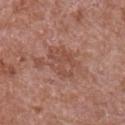Recorded during total-body skin imaging; not selected for excision or biopsy. Cropped from a total-body skin-imaging series; the visible field is about 15 mm. This is a white-light tile. On the right upper arm. The subject is a male aged 63 to 67. Automated image analysis of the tile measured an outline eccentricity of about 0.7 (0 = round, 1 = elongated) and a shape-asymmetry score of about 0.2 (0 = symmetric). And it measured a lesion color around L≈49 a*≈22 b*≈27 in CIELAB, about 7 CIELAB-L* units darker than the surrounding skin, and a normalized border contrast of about 5. The analysis additionally found a classifier nevus-likeness of about 0/100. Measured at roughly 4.5 mm in maximum diameter.